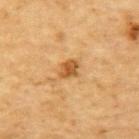Q: Was this lesion biopsied?
A: total-body-photography surveillance lesion; no biopsy
Q: What is the imaging modality?
A: total-body-photography crop, ~15 mm field of view
Q: Who is the patient?
A: male, roughly 85 years of age or older
Q: Lesion location?
A: the upper back